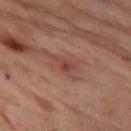follow-up: no biopsy performed (imaged during a skin exam) | image source: ~15 mm crop, total-body skin-cancer survey | subject: female, approximately 55 years of age | location: the left thigh | TBP lesion metrics: a border-irregularity rating of about 4.5/10, a color-variation rating of about 3/10, and a peripheral color-asymmetry measure near 1; an automated nevus-likeness rating near 0 out of 100 and a lesion-detection confidence of about 100/100 | tile lighting: cross-polarized illumination | lesion size: ≈3 mm.